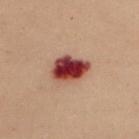Imaged during a routine full-body skin examination; the lesion was not biopsied and no histopathology is available. A female patient approximately 50 years of age. Cropped from a total-body skin-imaging series; the visible field is about 15 mm. From the chest.